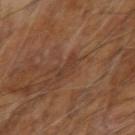Q: Was this lesion biopsied?
A: imaged on a skin check; not biopsied
Q: What is the anatomic site?
A: the arm
Q: Lesion size?
A: about 3 mm
Q: How was the tile lit?
A: cross-polarized
Q: Patient demographics?
A: male, aged approximately 60
Q: How was this image acquired?
A: ~15 mm crop, total-body skin-cancer survey